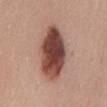Findings:
* workup · catalogued during a skin exam; not biopsied
* tile lighting · white-light
* lesion size · about 8 mm
* subject · female, aged 33–37
* body site · the chest
* acquisition · ~15 mm crop, total-body skin-cancer survey